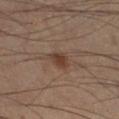Clinical impression: Captured during whole-body skin photography for melanoma surveillance; the lesion was not biopsied. Background: This is a cross-polarized tile. Automated image analysis of the tile measured an outline eccentricity of about 0.75 (0 = round, 1 = elongated) and a symmetry-axis asymmetry near 0.3. A male subject aged 53–57. The lesion is located on the right lower leg. A lesion tile, about 15 mm wide, cut from a 3D total-body photograph.